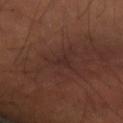No biopsy was performed on this lesion — it was imaged during a full skin examination and was not determined to be concerning.
A 15 mm close-up extracted from a 3D total-body photography capture.
The tile uses cross-polarized illumination.
Located on the left forearm.
Longest diameter approximately 3 mm.
A male patient, aged 48–52.